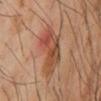Impression:
Recorded during total-body skin imaging; not selected for excision or biopsy.
Background:
A male patient aged around 60. On the chest. This is a cross-polarized tile. A 15 mm close-up tile from a total-body photography series done for melanoma screening. An algorithmic analysis of the crop reported a lesion area of about 15 mm², an outline eccentricity of about 0.85 (0 = round, 1 = elongated), and a shape-asymmetry score of about 0.35 (0 = symmetric). And it measured an average lesion color of about L≈45 a*≈22 b*≈30 (CIELAB), a lesion–skin lightness drop of about 9, and a lesion-to-skin contrast of about 7.5 (normalized; higher = more distinct). It also reported border irregularity of about 4 on a 0–10 scale, internal color variation of about 8 on a 0–10 scale, and peripheral color asymmetry of about 3. It also reported an automated nevus-likeness rating near 10 out of 100 and a lesion-detection confidence of about 100/100.